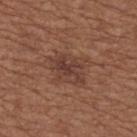Assessment: Imaged during a routine full-body skin examination; the lesion was not biopsied and no histopathology is available. Background: Captured under white-light illumination. Automated image analysis of the tile measured a lesion color around L≈38 a*≈20 b*≈25 in CIELAB, roughly 8 lightness units darker than nearby skin, and a normalized lesion–skin contrast near 7.5. It also reported a border-irregularity rating of about 6/10 and peripheral color asymmetry of about 1. The software also gave a lesion-detection confidence of about 100/100. The recorded lesion diameter is about 4 mm. On the upper back. Cropped from a whole-body photographic skin survey; the tile spans about 15 mm. A female subject, aged around 65.